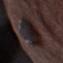Part of a total-body skin-imaging series; this lesion was reviewed on a skin check and was not flagged for biopsy. The lesion is on the left thigh. A male subject in their mid- to late 70s. A 15 mm crop from a total-body photograph taken for skin-cancer surveillance. Measured at roughly 8.5 mm in maximum diameter. An algorithmic analysis of the crop reported a border-irregularity rating of about 4/10, a within-lesion color-variation index near 9/10, and radial color variation of about 3. Imaged with white-light lighting.